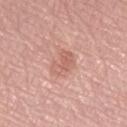Acquisition and patient details:
A female patient, roughly 65 years of age. This is a white-light tile. The lesion is located on the right forearm. Longest diameter approximately 3 mm. Cropped from a total-body skin-imaging series; the visible field is about 15 mm.